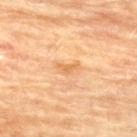notes = no biopsy performed (imaged during a skin exam)
site = the back
subject = female, aged approximately 80
image = ~15 mm tile from a whole-body skin photo
size = about 2.5 mm